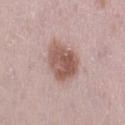  image:
    source: total-body photography crop
    field_of_view_mm: 15
  site: leg
  patient:
    sex: female
    age_approx: 30
  lighting: white-light